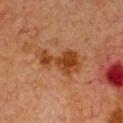Clinical summary:
The subject is a female aged 38–42. A 15 mm close-up extracted from a 3D total-body photography capture. Automated image analysis of the tile measured a footprint of about 14 mm² and a shape eccentricity near 0.9. And it measured a lesion–skin lightness drop of about 9 and a lesion-to-skin contrast of about 8.5 (normalized; higher = more distinct). It also reported a border-irregularity index near 5/10 and a color-variation rating of about 4/10. The lesion is located on the front of the torso. Captured under cross-polarized illumination.